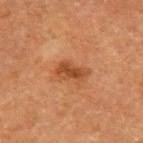No biopsy was performed on this lesion — it was imaged during a full skin examination and was not determined to be concerning. Cropped from a total-body skin-imaging series; the visible field is about 15 mm. The lesion is located on the left upper arm. A male subject, aged around 75.